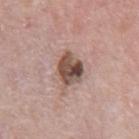notes — total-body-photography surveillance lesion; no biopsy
image — total-body-photography crop, ~15 mm field of view
lesion size — about 4.5 mm
illumination — white-light illumination
image-analysis metrics — a footprint of about 9.5 mm², an outline eccentricity of about 0.65 (0 = round, 1 = elongated), and a symmetry-axis asymmetry near 0.25; a detector confidence of about 100 out of 100 that the crop contains a lesion
site — the chest
subject — male, in their 70s